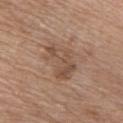biopsy_status: not biopsied; imaged during a skin examination
lesion_size:
  long_diameter_mm_approx: 5.0
image:
  source: total-body photography crop
  field_of_view_mm: 15
site: front of the torso
automated_metrics:
  eccentricity: 0.75
  shape_asymmetry: 0.35
  cielab_L: 51
  cielab_a: 17
  cielab_b: 28
  vs_skin_darker_L: 8.0
  vs_skin_contrast_norm: 5.5
patient:
  sex: male
  age_approx: 70
lighting: white-light